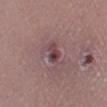No biopsy was performed on this lesion — it was imaged during a full skin examination and was not determined to be concerning. On the left lower leg. The patient is a female in their 40s. A roughly 15 mm field-of-view crop from a total-body skin photograph. About 2.5 mm across.